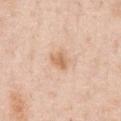Captured during whole-body skin photography for melanoma surveillance; the lesion was not biopsied. This is a white-light tile. Automated tile analysis of the lesion measured an area of roughly 4 mm², an eccentricity of roughly 0.55, and a shape-asymmetry score of about 0.3 (0 = symmetric). The patient is a male roughly 50 years of age. From the chest. A 15 mm crop from a total-body photograph taken for skin-cancer surveillance.